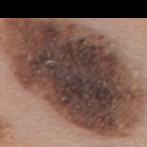Part of a total-body skin-imaging series; this lesion was reviewed on a skin check and was not flagged for biopsy. A region of skin cropped from a whole-body photographic capture, roughly 15 mm wide. Measured at roughly 17.5 mm in maximum diameter. The total-body-photography lesion software estimated a footprint of about 105 mm² and a shape-asymmetry score of about 0.15 (0 = symmetric). The software also gave border irregularity of about 3 on a 0–10 scale, a within-lesion color-variation index near 8.5/10, and peripheral color asymmetry of about 2.5. The subject is a female aged 58–62. On the back.